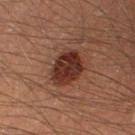notes: total-body-photography surveillance lesion; no biopsy | tile lighting: cross-polarized | location: the right thigh | subject: male, aged around 40 | TBP lesion metrics: a shape eccentricity near 0.6; lesion-presence confidence of about 100/100 | imaging modality: 15 mm crop, total-body photography.